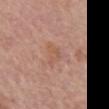Clinical impression:
The lesion was photographed on a routine skin check and not biopsied; there is no pathology result.
Context:
A 15 mm close-up extracted from a 3D total-body photography capture. A female subject about 75 years old. On the mid back. Imaged with white-light lighting.